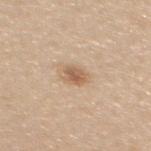Recorded during total-body skin imaging; not selected for excision or biopsy.
The tile uses white-light illumination.
A 15 mm crop from a total-body photograph taken for skin-cancer surveillance.
Approximately 2.5 mm at its widest.
On the upper back.
The patient is a female aged approximately 25.